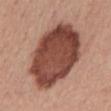Findings:
* follow-up — catalogued during a skin exam; not biopsied
* imaging modality — total-body-photography crop, ~15 mm field of view
* location — the mid back
* subject — female, about 55 years old